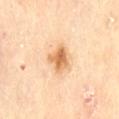  biopsy_status: not biopsied; imaged during a skin examination
  image:
    source: total-body photography crop
    field_of_view_mm: 15
  site: lower back
  patient:
    sex: female
    age_approx: 60